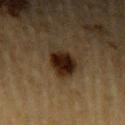biopsy status — catalogued during a skin exam; not biopsied
subject — male, about 85 years old or older
illumination — cross-polarized
image — ~15 mm crop, total-body skin-cancer survey
body site — the left upper arm
diameter — ≈4.5 mm